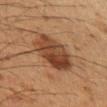Findings:
• biopsy status · imaged on a skin check; not biopsied
• body site · the arm
• acquisition · ~15 mm crop, total-body skin-cancer survey
• subject · male, in their 50s
• lesion size · ≈6 mm
• lighting · cross-polarized
• automated lesion analysis · a footprint of about 18 mm², an outline eccentricity of about 0.8 (0 = round, 1 = elongated), and a symmetry-axis asymmetry near 0.15; a mean CIELAB color near L≈38 a*≈20 b*≈30, about 11 CIELAB-L* units darker than the surrounding skin, and a normalized border contrast of about 9.5; a border-irregularity index near 2/10, a color-variation rating of about 6.5/10, and a peripheral color-asymmetry measure near 2.5; a classifier nevus-likeness of about 65/100 and lesion-presence confidence of about 100/100